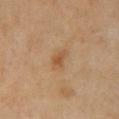This lesion was catalogued during total-body skin photography and was not selected for biopsy. Cropped from a whole-body photographic skin survey; the tile spans about 15 mm. Located on the chest. The subject is a female approximately 55 years of age. Captured under cross-polarized illumination.